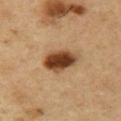Assessment:
This lesion was catalogued during total-body skin photography and was not selected for biopsy.
Background:
A male patient approximately 55 years of age. Imaged with cross-polarized lighting. About 4.5 mm across. On the chest. A 15 mm close-up extracted from a 3D total-body photography capture.